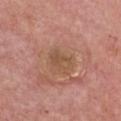The lesion was photographed on a routine skin check and not biopsied; there is no pathology result.
Captured under white-light illumination.
A female patient, aged 63 to 67.
Longest diameter approximately 3 mm.
From the front of the torso.
Cropped from a total-body skin-imaging series; the visible field is about 15 mm.
An algorithmic analysis of the crop reported an average lesion color of about L≈51 a*≈20 b*≈31 (CIELAB), about 7 CIELAB-L* units darker than the surrounding skin, and a normalized border contrast of about 5.